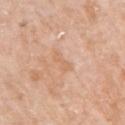The lesion was photographed on a routine skin check and not biopsied; there is no pathology result. A female subject roughly 75 years of age. Automated image analysis of the tile measured a lesion area of about 3 mm² and an eccentricity of roughly 0.9. The analysis additionally found an average lesion color of about L≈65 a*≈21 b*≈35 (CIELAB), roughly 6 lightness units darker than nearby skin, and a normalized lesion–skin contrast near 4.5. It also reported a border-irregularity index near 5/10, internal color variation of about 0 on a 0–10 scale, and peripheral color asymmetry of about 0. Imaged with white-light lighting. A lesion tile, about 15 mm wide, cut from a 3D total-body photograph. About 2.5 mm across. On the left upper arm.